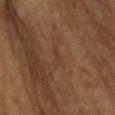follow-up = total-body-photography surveillance lesion; no biopsy | site = the head or neck | lesion diameter = about 2 mm | subject = male, in their 70s | image = total-body-photography crop, ~15 mm field of view.